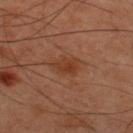Assessment:
Captured during whole-body skin photography for melanoma surveillance; the lesion was not biopsied.
Acquisition and patient details:
Captured under cross-polarized illumination. A male patient, in their mid-40s. Longest diameter approximately 3.5 mm. Located on the upper back. A roughly 15 mm field-of-view crop from a total-body skin photograph.